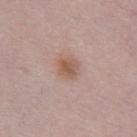Imaged during a routine full-body skin examination; the lesion was not biopsied and no histopathology is available.
A female patient, aged 53 to 57.
On the chest.
The tile uses white-light illumination.
A region of skin cropped from a whole-body photographic capture, roughly 15 mm wide.